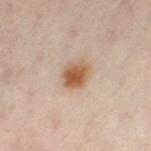The lesion was photographed on a routine skin check and not biopsied; there is no pathology result.
Located on the right leg.
A close-up tile cropped from a whole-body skin photograph, about 15 mm across.
The subject is a female roughly 40 years of age.